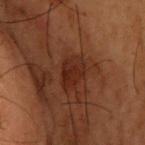Q: Was this lesion biopsied?
A: imaged on a skin check; not biopsied
Q: What lighting was used for the tile?
A: cross-polarized
Q: Lesion size?
A: ~3.5 mm (longest diameter)
Q: Patient demographics?
A: male, aged approximately 55
Q: Automated lesion metrics?
A: a mean CIELAB color near L≈21 a*≈20 b*≈24 and a lesion–skin lightness drop of about 6; a border-irregularity rating of about 2.5/10, internal color variation of about 2.5 on a 0–10 scale, and a peripheral color-asymmetry measure near 1; an automated nevus-likeness rating near 25 out of 100 and a detector confidence of about 100 out of 100 that the crop contains a lesion
Q: What is the imaging modality?
A: total-body-photography crop, ~15 mm field of view
Q: What is the anatomic site?
A: the upper back